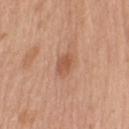Part of a total-body skin-imaging series; this lesion was reviewed on a skin check and was not flagged for biopsy. A female patient, roughly 75 years of age. The lesion is located on the left upper arm. Longest diameter approximately 2.5 mm. A 15 mm close-up tile from a total-body photography series done for melanoma screening. Captured under white-light illumination.